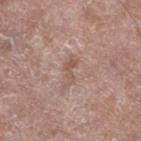| feature | finding |
|---|---|
| follow-up | no biopsy performed (imaged during a skin exam) |
| body site | the left lower leg |
| patient | male, aged approximately 65 |
| acquisition | 15 mm crop, total-body photography |